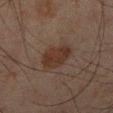<case>
  <biopsy_status>not biopsied; imaged during a skin examination</biopsy_status>
  <patient>
    <sex>male</sex>
    <age_approx>45</age_approx>
  </patient>
  <site>right lower leg</site>
  <automated_metrics>
    <area_mm2_approx>9.0</area_mm2_approx>
    <eccentricity>0.65</eccentricity>
    <shape_asymmetry>0.15</shape_asymmetry>
    <cielab_L>26</cielab_L>
    <cielab_a>14</cielab_a>
    <cielab_b>20</cielab_b>
    <vs_skin_darker_L>7.0</vs_skin_darker_L>
    <color_variation_0_10>2.0</color_variation_0_10>
    <peripheral_color_asymmetry>0.5</peripheral_color_asymmetry>
  </automated_metrics>
  <image>
    <source>total-body photography crop</source>
    <field_of_view_mm>15</field_of_view_mm>
  </image>
  <lesion_size>
    <long_diameter_mm_approx>4.0</long_diameter_mm_approx>
  </lesion_size>
</case>